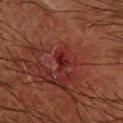Assessment: Captured during whole-body skin photography for melanoma surveillance; the lesion was not biopsied. Clinical summary: A male subject about 65 years old. The lesion is on the arm. A lesion tile, about 15 mm wide, cut from a 3D total-body photograph. This is a cross-polarized tile. The lesion's longest dimension is about 2.5 mm.